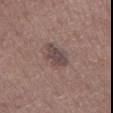biopsy status: total-body-photography surveillance lesion; no biopsy
site: the front of the torso
imaging modality: ~15 mm crop, total-body skin-cancer survey
subject: male, aged 58–62
lesion diameter: about 4.5 mm
image-analysis metrics: a mean CIELAB color near L≈45 a*≈16 b*≈18, about 10 CIELAB-L* units darker than the surrounding skin, and a normalized lesion–skin contrast near 8; border irregularity of about 4 on a 0–10 scale and a within-lesion color-variation index near 3/10; a classifier nevus-likeness of about 0/100 and a detector confidence of about 100 out of 100 that the crop contains a lesion